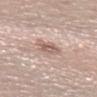<record>
  <biopsy_status>not biopsied; imaged during a skin examination</biopsy_status>
  <lesion_size>
    <long_diameter_mm_approx>3.0</long_diameter_mm_approx>
  </lesion_size>
  <lighting>white-light</lighting>
  <site>left forearm</site>
  <image>
    <source>total-body photography crop</source>
    <field_of_view_mm>15</field_of_view_mm>
  </image>
  <patient>
    <sex>female</sex>
    <age_approx>40</age_approx>
  </patient>
</record>